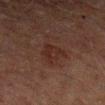Captured during whole-body skin photography for melanoma surveillance; the lesion was not biopsied. An algorithmic analysis of the crop reported a mean CIELAB color near L≈20 a*≈16 b*≈18 and a normalized border contrast of about 6. And it measured a nevus-likeness score of about 0/100. On the left forearm. A male subject, in their 60s. A close-up tile cropped from a whole-body skin photograph, about 15 mm across. Imaged with cross-polarized lighting.